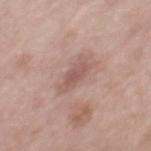Clinical impression:
Imaged during a routine full-body skin examination; the lesion was not biopsied and no histopathology is available.
Image and clinical context:
A male subject in their mid-50s. Automated image analysis of the tile measured a lesion color around L≈56 a*≈19 b*≈23 in CIELAB and a normalized lesion–skin contrast near 6. The analysis additionally found a border-irregularity index near 3.5/10 and a color-variation rating of about 2/10. It also reported an automated nevus-likeness rating near 0 out of 100. Located on the mid back. Measured at roughly 4 mm in maximum diameter. This image is a 15 mm lesion crop taken from a total-body photograph. Imaged with white-light lighting.